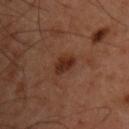– notes: no biopsy performed (imaged during a skin exam)
– tile lighting: cross-polarized illumination
– subject: male, in their mid- to late 40s
– acquisition: total-body-photography crop, ~15 mm field of view
– body site: the chest
– diameter: ≈3 mm